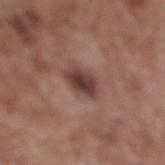{
  "biopsy_status": "not biopsied; imaged during a skin examination",
  "site": "leg",
  "patient": {
    "sex": "male",
    "age_approx": 60
  },
  "automated_metrics": {
    "eccentricity": 0.65,
    "cielab_L": 40,
    "cielab_a": 19,
    "cielab_b": 21,
    "vs_skin_darker_L": 13.0,
    "vs_skin_contrast_norm": 10.0,
    "color_variation_0_10": 4.0,
    "peripheral_color_asymmetry": 1.0,
    "nevus_likeness_0_100": 25
  },
  "image": {
    "source": "total-body photography crop",
    "field_of_view_mm": 15
  },
  "lesion_size": {
    "long_diameter_mm_approx": 3.0
  },
  "lighting": "white-light"
}